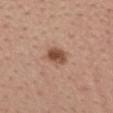notes: no biopsy performed (imaged during a skin exam)
image-analysis metrics: an eccentricity of roughly 0.75 and a shape-asymmetry score of about 0.15 (0 = symmetric); a lesion color around L≈50 a*≈21 b*≈29 in CIELAB, roughly 13 lightness units darker than nearby skin, and a normalized border contrast of about 9.5; an automated nevus-likeness rating near 95 out of 100 and lesion-presence confidence of about 100/100
body site: the mid back
subject: female, roughly 55 years of age
image: 15 mm crop, total-body photography
lesion diameter: ~3 mm (longest diameter)
tile lighting: white-light